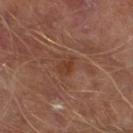Q: Is there a histopathology result?
A: imaged on a skin check; not biopsied
Q: What is the anatomic site?
A: the left lower leg
Q: Lesion size?
A: about 2.5 mm
Q: How was the tile lit?
A: cross-polarized illumination
Q: What kind of image is this?
A: 15 mm crop, total-body photography
Q: Who is the patient?
A: male, in their mid- to late 60s